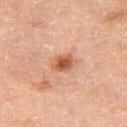Part of a total-body skin-imaging series; this lesion was reviewed on a skin check and was not flagged for biopsy. The tile uses cross-polarized illumination. A 15 mm close-up extracted from a 3D total-body photography capture. Located on the leg. An algorithmic analysis of the crop reported a lesion area of about 6 mm², an eccentricity of roughly 0.7, and two-axis asymmetry of about 0.2. A female patient roughly 55 years of age.